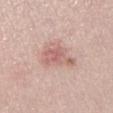workup = no biopsy performed (imaged during a skin exam); size = ≈5 mm; subject = male, about 30 years old; lighting = white-light; image = ~15 mm tile from a whole-body skin photo.